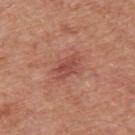| key | value |
|---|---|
| biopsy status | catalogued during a skin exam; not biopsied |